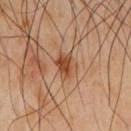Recorded during total-body skin imaging; not selected for excision or biopsy. Imaged with cross-polarized lighting. The lesion is located on the front of the torso. A male subject, aged 63–67. Measured at roughly 3 mm in maximum diameter. A lesion tile, about 15 mm wide, cut from a 3D total-body photograph.